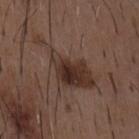| feature | finding |
|---|---|
| biopsy status | no biopsy performed (imaged during a skin exam) |